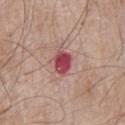biopsy status = total-body-photography surveillance lesion; no biopsy
patient = male, aged 63–67
image = 15 mm crop, total-body photography
tile lighting = white-light illumination
anatomic site = the chest
diameter = ~3 mm (longest diameter)